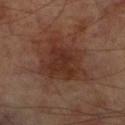Part of a total-body skin-imaging series; this lesion was reviewed on a skin check and was not flagged for biopsy.
A close-up tile cropped from a whole-body skin photograph, about 15 mm across.
Automated tile analysis of the lesion measured an area of roughly 22 mm² and a shape-asymmetry score of about 0.3 (0 = symmetric). The software also gave a lesion color around L≈31 a*≈20 b*≈25 in CIELAB and roughly 8 lightness units darker than nearby skin. And it measured a border-irregularity index near 4/10, a within-lesion color-variation index near 4/10, and radial color variation of about 1.5. It also reported a nevus-likeness score of about 15/100 and a detector confidence of about 100 out of 100 that the crop contains a lesion.
This is a cross-polarized tile.
A male patient, aged 68 to 72.
The lesion is on the leg.